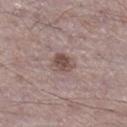{"biopsy_status": "not biopsied; imaged during a skin examination", "automated_metrics": {"cielab_L": 49, "cielab_a": 17, "cielab_b": 20, "vs_skin_contrast_norm": 8.0, "border_irregularity_0_10": 2.0, "color_variation_0_10": 3.5, "peripheral_color_asymmetry": 1.5, "nevus_likeness_0_100": 85, "lesion_detection_confidence_0_100": 100}, "lighting": "white-light", "patient": {"sex": "male", "age_approx": 50}, "lesion_size": {"long_diameter_mm_approx": 2.5}, "site": "right lower leg", "image": {"source": "total-body photography crop", "field_of_view_mm": 15}}